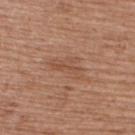No biopsy was performed on this lesion — it was imaged during a full skin examination and was not determined to be concerning. The lesion is located on the upper back. A 15 mm crop from a total-body photograph taken for skin-cancer surveillance. The subject is a female aged approximately 40.